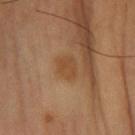Impression:
No biopsy was performed on this lesion — it was imaged during a full skin examination and was not determined to be concerning.
Context:
A male subject roughly 60 years of age. This is a cross-polarized tile. Cropped from a total-body skin-imaging series; the visible field is about 15 mm. The lesion's longest dimension is about 3 mm. On the left forearm.